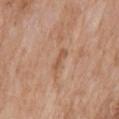Imaged during a routine full-body skin examination; the lesion was not biopsied and no histopathology is available. Located on the mid back. This image is a 15 mm lesion crop taken from a total-body photograph. The subject is a female roughly 75 years of age.